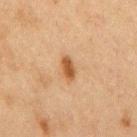Notes:
- follow-up · imaged on a skin check; not biopsied
- lesion diameter · ~3 mm (longest diameter)
- subject · male, aged 73 to 77
- lighting · cross-polarized illumination
- site · the mid back
- automated metrics · an average lesion color of about L≈44 a*≈19 b*≈33 (CIELAB), roughly 11 lightness units darker than nearby skin, and a normalized lesion–skin contrast near 9
- image · ~15 mm tile from a whole-body skin photo